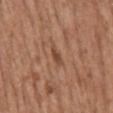workup — catalogued during a skin exam; not biopsied | image-analysis metrics — a footprint of about 3 mm², an outline eccentricity of about 0.9 (0 = round, 1 = elongated), and a symmetry-axis asymmetry near 0.25; a lesion color around L≈47 a*≈21 b*≈31 in CIELAB and about 8 CIELAB-L* units darker than the surrounding skin; a border-irregularity index near 2.5/10, internal color variation of about 1 on a 0–10 scale, and peripheral color asymmetry of about 0; a lesion-detection confidence of about 100/100 | lighting — white-light | subject — female, aged 63 to 67 | body site — the mid back | image — total-body-photography crop, ~15 mm field of view.